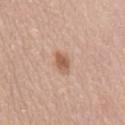Image and clinical context:
Located on the mid back. A region of skin cropped from a whole-body photographic capture, roughly 15 mm wide. The subject is a female aged 48–52.